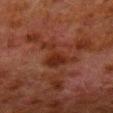{
  "biopsy_status": "not biopsied; imaged during a skin examination",
  "site": "left lower leg",
  "lighting": "cross-polarized",
  "image": {
    "source": "total-body photography crop",
    "field_of_view_mm": 15
  },
  "automated_metrics": {
    "eccentricity": 0.85,
    "shape_asymmetry": 0.7,
    "cielab_L": 23,
    "cielab_a": 21,
    "cielab_b": 24,
    "vs_skin_darker_L": 6.0,
    "vs_skin_contrast_norm": 7.5,
    "border_irregularity_0_10": 8.5,
    "color_variation_0_10": 1.5,
    "peripheral_color_asymmetry": 0.5,
    "nevus_likeness_0_100": 0,
    "lesion_detection_confidence_0_100": 100
  },
  "patient": {
    "sex": "male",
    "age_approx": 80
  },
  "lesion_size": {
    "long_diameter_mm_approx": 5.0
  }
}